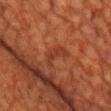Q: Was this lesion biopsied?
A: catalogued during a skin exam; not biopsied
Q: Lesion location?
A: the chest
Q: What lighting was used for the tile?
A: cross-polarized
Q: What are the patient's age and sex?
A: male, approximately 60 years of age
Q: What is the lesion's diameter?
A: ≈3.5 mm
Q: How was this image acquired?
A: total-body-photography crop, ~15 mm field of view